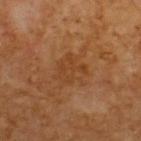Clinical impression:
No biopsy was performed on this lesion — it was imaged during a full skin examination and was not determined to be concerning.
Background:
The tile uses cross-polarized illumination. Longest diameter approximately 3.5 mm. Cropped from a total-body skin-imaging series; the visible field is about 15 mm. Located on the upper back. The lesion-visualizer software estimated an area of roughly 8.5 mm² and a shape-asymmetry score of about 0.35 (0 = symmetric). It also reported a mean CIELAB color near L≈38 a*≈20 b*≈33, a lesion–skin lightness drop of about 5, and a normalized lesion–skin contrast near 5. And it measured border irregularity of about 4 on a 0–10 scale and peripheral color asymmetry of about 1. The patient is a male about 60 years old.